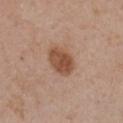{
  "biopsy_status": "not biopsied; imaged during a skin examination",
  "lesion_size": {
    "long_diameter_mm_approx": 4.0
  },
  "automated_metrics": {
    "cielab_L": 50,
    "cielab_a": 21,
    "cielab_b": 31,
    "vs_skin_darker_L": 12.0,
    "vs_skin_contrast_norm": 9.0,
    "color_variation_0_10": 3.5,
    "peripheral_color_asymmetry": 1.0
  },
  "patient": {
    "sex": "female",
    "age_approx": 55
  },
  "site": "chest",
  "image": {
    "source": "total-body photography crop",
    "field_of_view_mm": 15
  }
}